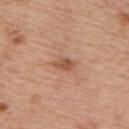Q: Was a biopsy performed?
A: total-body-photography surveillance lesion; no biopsy
Q: What is the imaging modality?
A: ~15 mm crop, total-body skin-cancer survey
Q: Illumination type?
A: white-light
Q: Patient demographics?
A: male, in their mid- to late 70s
Q: How large is the lesion?
A: ≈3 mm
Q: What is the anatomic site?
A: the upper back